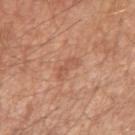Clinical impression: This lesion was catalogued during total-body skin photography and was not selected for biopsy. Background: Automated image analysis of the tile measured a footprint of about 4.5 mm², an eccentricity of roughly 0.9, and a symmetry-axis asymmetry near 0.25. The software also gave roughly 7 lightness units darker than nearby skin and a lesion-to-skin contrast of about 5 (normalized; higher = more distinct). The analysis additionally found border irregularity of about 3 on a 0–10 scale, a within-lesion color-variation index near 2/10, and peripheral color asymmetry of about 0.5. And it measured a classifier nevus-likeness of about 0/100 and lesion-presence confidence of about 100/100. A male patient, about 65 years old. The lesion is on the right upper arm. Measured at roughly 3.5 mm in maximum diameter. This is a white-light tile. A 15 mm crop from a total-body photograph taken for skin-cancer surveillance.